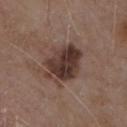workup = imaged on a skin check; not biopsied
location = the chest
patient = male, aged around 80
lighting = white-light illumination
imaging modality = total-body-photography crop, ~15 mm field of view